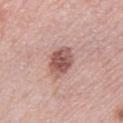follow-up: no biopsy performed (imaged during a skin exam) | image: ~15 mm crop, total-body skin-cancer survey | subject: female, aged approximately 65 | tile lighting: white-light illumination | lesion diameter: ~4 mm (longest diameter) | body site: the leg.